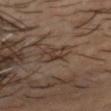Context:
This image is a 15 mm lesion crop taken from a total-body photograph. Captured under cross-polarized illumination. The lesion is located on the head or neck. A male subject roughly 50 years of age. The total-body-photography lesion software estimated a shape-asymmetry score of about 0.25 (0 = symmetric). And it measured a nevus-likeness score of about 0/100 and a lesion-detection confidence of about 95/100.